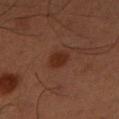Clinical impression: The lesion was tiled from a total-body skin photograph and was not biopsied. Context: A 15 mm close-up extracted from a 3D total-body photography capture. The patient is a male in their 50s. The lesion is located on the left lower leg.